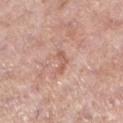| key | value |
|---|---|
| acquisition | ~15 mm crop, total-body skin-cancer survey |
| subject | male, aged 73 to 77 |
| automated lesion analysis | a footprint of about 2.5 mm² and an outline eccentricity of about 0.9 (0 = round, 1 = elongated); a lesion color around L≈59 a*≈23 b*≈29 in CIELAB and a lesion–skin lightness drop of about 8; an automated nevus-likeness rating near 0 out of 100 and lesion-presence confidence of about 100/100 |
| location | the left lower leg |
| diameter | ~3 mm (longest diameter) |